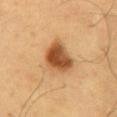This lesion was catalogued during total-body skin photography and was not selected for biopsy.
A male patient, aged around 60.
From the lower back.
This image is a 15 mm lesion crop taken from a total-body photograph.
An algorithmic analysis of the crop reported an outline eccentricity of about 0.6 (0 = round, 1 = elongated) and a shape-asymmetry score of about 0.25 (0 = symmetric). And it measured a lesion color around L≈51 a*≈24 b*≈40 in CIELAB, a lesion–skin lightness drop of about 17, and a normalized border contrast of about 11. And it measured a border-irregularity index near 2.5/10 and peripheral color asymmetry of about 1.5. The analysis additionally found an automated nevus-likeness rating near 100 out of 100.
Captured under cross-polarized illumination.
The lesion's longest dimension is about 4 mm.